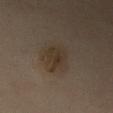Findings:
- notes · catalogued during a skin exam; not biopsied
- imaging modality · total-body-photography crop, ~15 mm field of view
- anatomic site · the arm
- patient · male, aged approximately 55
- lighting · cross-polarized
- lesion size · about 4 mm